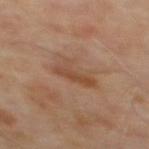follow-up: imaged on a skin check; not biopsied | subject: male, about 65 years old | acquisition: ~15 mm crop, total-body skin-cancer survey | body site: the back | illumination: cross-polarized | image-analysis metrics: a mean CIELAB color near L≈45 a*≈20 b*≈31, about 7 CIELAB-L* units darker than the surrounding skin, and a normalized lesion–skin contrast near 6.5; internal color variation of about 3.5 on a 0–10 scale and radial color variation of about 1.